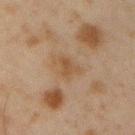Assessment: Recorded during total-body skin imaging; not selected for excision or biopsy. Clinical summary: About 3 mm across. A region of skin cropped from a whole-body photographic capture, roughly 15 mm wide. The lesion-visualizer software estimated a lesion area of about 3.5 mm² and two-axis asymmetry of about 0.4. The analysis additionally found a mean CIELAB color near L≈40 a*≈15 b*≈28 and about 6 CIELAB-L* units darker than the surrounding skin. It also reported a border-irregularity rating of about 4.5/10, a within-lesion color-variation index near 1.5/10, and a peripheral color-asymmetry measure near 0.5. The patient is a male aged approximately 45. This is a cross-polarized tile. On the arm.